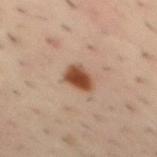Q: Illumination type?
A: cross-polarized
Q: Where on the body is the lesion?
A: the mid back
Q: How was this image acquired?
A: total-body-photography crop, ~15 mm field of view
Q: Lesion size?
A: ≈3 mm
Q: What are the patient's age and sex?
A: male, in their 40s